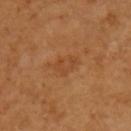The lesion was photographed on a routine skin check and not biopsied; there is no pathology result. A region of skin cropped from a whole-body photographic capture, roughly 15 mm wide. This is a cross-polarized tile. The lesion is on the left upper arm. Longest diameter approximately 4 mm. A female patient aged 53–57.